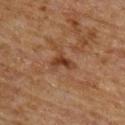<tbp_lesion>
  <biopsy_status>not biopsied; imaged during a skin examination</biopsy_status>
</tbp_lesion>